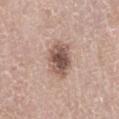Image and clinical context: On the abdomen. An algorithmic analysis of the crop reported an area of roughly 12 mm², an outline eccentricity of about 0.55 (0 = round, 1 = elongated), and a shape-asymmetry score of about 0.2 (0 = symmetric). The software also gave a lesion color around L≈54 a*≈18 b*≈24 in CIELAB, roughly 14 lightness units darker than nearby skin, and a normalized lesion–skin contrast near 9.5. And it measured a classifier nevus-likeness of about 60/100. Cropped from a whole-body photographic skin survey; the tile spans about 15 mm. A female subject roughly 60 years of age. Captured under white-light illumination.